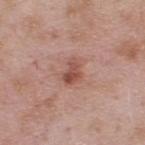workup = no biopsy performed (imaged during a skin exam); patient = male, aged 53 to 57; body site = the upper back; lesion diameter = about 3 mm; image source = ~15 mm crop, total-body skin-cancer survey.